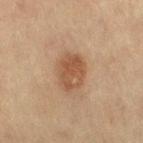<case>
<biopsy_status>not biopsied; imaged during a skin examination</biopsy_status>
<image>
  <source>total-body photography crop</source>
  <field_of_view_mm>15</field_of_view_mm>
</image>
<lighting>cross-polarized</lighting>
<patient>
  <sex>female</sex>
  <age_approx>55</age_approx>
</patient>
<lesion_size>
  <long_diameter_mm_approx>4.0</long_diameter_mm_approx>
</lesion_size>
<site>right thigh</site>
<automated_metrics>
  <area_mm2_approx>9.5</area_mm2_approx>
  <eccentricity>0.7</eccentricity>
  <nevus_likeness_0_100>95</nevus_likeness_0_100>
  <lesion_detection_confidence_0_100>100</lesion_detection_confidence_0_100>
</automated_metrics>
</case>